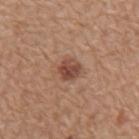Longest diameter approximately 2.5 mm. The lesion is on the mid back. Automated image analysis of the tile measured an area of roughly 5 mm², an eccentricity of roughly 0.4, and a shape-asymmetry score of about 0.25 (0 = symmetric). And it measured about 12 CIELAB-L* units darker than the surrounding skin. And it measured internal color variation of about 3.5 on a 0–10 scale and radial color variation of about 1.5. It also reported a classifier nevus-likeness of about 90/100 and a lesion-detection confidence of about 100/100. A 15 mm crop from a total-body photograph taken for skin-cancer surveillance. A male subject, in their mid-60s.